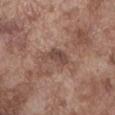Imaged during a routine full-body skin examination; the lesion was not biopsied and no histopathology is available.
From the front of the torso.
Automated tile analysis of the lesion measured an average lesion color of about L≈45 a*≈18 b*≈23 (CIELAB) and a lesion–skin lightness drop of about 9. And it measured border irregularity of about 4 on a 0–10 scale, a color-variation rating of about 3/10, and a peripheral color-asymmetry measure near 1.
Approximately 3.5 mm at its widest.
The patient is a male aged approximately 75.
A 15 mm crop from a total-body photograph taken for skin-cancer surveillance.
This is a white-light tile.